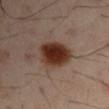Captured during whole-body skin photography for melanoma surveillance; the lesion was not biopsied. Captured under cross-polarized illumination. A male patient about 50 years old. A 15 mm close-up tile from a total-body photography series done for melanoma screening. Automated image analysis of the tile measured an area of roughly 14 mm² and an eccentricity of roughly 0.7. The analysis additionally found a classifier nevus-likeness of about 100/100 and lesion-presence confidence of about 100/100. Approximately 5 mm at its widest. The lesion is located on the left upper arm.